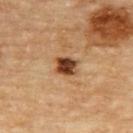Image and clinical context:
This is a cross-polarized tile. Longest diameter approximately 3 mm. The total-body-photography lesion software estimated an area of roughly 5.5 mm², a shape eccentricity near 0.55, and two-axis asymmetry of about 0.2. The analysis additionally found a lesion color around L≈42 a*≈22 b*≈34 in CIELAB, roughly 18 lightness units darker than nearby skin, and a normalized border contrast of about 13. And it measured a border-irregularity rating of about 2/10, a color-variation rating of about 5/10, and peripheral color asymmetry of about 1.5. And it measured a nevus-likeness score of about 95/100. The lesion is on the upper back. Cropped from a whole-body photographic skin survey; the tile spans about 15 mm. A male patient, aged 83 or over.